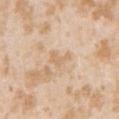Imaged during a routine full-body skin examination; the lesion was not biopsied and no histopathology is available. A 15 mm crop from a total-body photograph taken for skin-cancer surveillance. Located on the left upper arm. Automated tile analysis of the lesion measured a footprint of about 3 mm², an eccentricity of roughly 0.8, and a shape-asymmetry score of about 0.55 (0 = symmetric). The analysis additionally found an average lesion color of about L≈68 a*≈16 b*≈35 (CIELAB), about 7 CIELAB-L* units darker than the surrounding skin, and a lesion-to-skin contrast of about 5 (normalized; higher = more distinct). Captured under white-light illumination. Longest diameter approximately 2.5 mm. A female subject, about 25 years old.